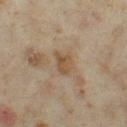– workup — total-body-photography surveillance lesion; no biopsy
– illumination — cross-polarized illumination
– body site — the leg
– image — ~15 mm crop, total-body skin-cancer survey
– patient — female, about 35 years old
– automated lesion analysis — an automated nevus-likeness rating near 0 out of 100 and lesion-presence confidence of about 100/100
– size — ~2.5 mm (longest diameter)